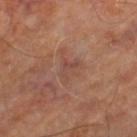notes: imaged on a skin check; not biopsied | imaging modality: total-body-photography crop, ~15 mm field of view | site: the leg | subject: aged 63–67.